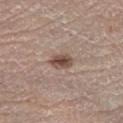{"biopsy_status": "not biopsied; imaged during a skin examination", "site": "leg", "lighting": "white-light", "image": {"source": "total-body photography crop", "field_of_view_mm": 15}, "patient": {"sex": "female", "age_approx": 60}, "automated_metrics": {"eccentricity": 0.75, "cielab_L": 48, "cielab_a": 17, "cielab_b": 24, "vs_skin_darker_L": 13.0, "vs_skin_contrast_norm": 9.5}}